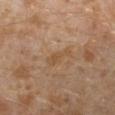Approximately 3.5 mm at its widest.
Imaged with cross-polarized lighting.
The lesion is on the left lower leg.
A male subject, aged approximately 30.
A 15 mm close-up extracted from a 3D total-body photography capture.